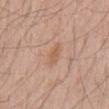Clinical impression: Part of a total-body skin-imaging series; this lesion was reviewed on a skin check and was not flagged for biopsy. Background: The lesion is located on the mid back. Longest diameter approximately 3 mm. Captured under white-light illumination. This image is a 15 mm lesion crop taken from a total-body photograph. An algorithmic analysis of the crop reported an area of roughly 3 mm² and a shape eccentricity near 0.9. The analysis additionally found an average lesion color of about L≈59 a*≈20 b*≈32 (CIELAB), about 7 CIELAB-L* units darker than the surrounding skin, and a normalized lesion–skin contrast near 5.5. A male patient approximately 60 years of age.